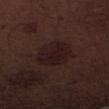Part of a total-body skin-imaging series; this lesion was reviewed on a skin check and was not flagged for biopsy.
A 15 mm close-up tile from a total-body photography series done for melanoma screening.
The total-body-photography lesion software estimated a lesion area of about 14 mm², an outline eccentricity of about 0.65 (0 = round, 1 = elongated), and two-axis asymmetry of about 0.15. It also reported a classifier nevus-likeness of about 25/100 and a detector confidence of about 100 out of 100 that the crop contains a lesion.
The patient is a male aged around 70.
Located on the abdomen.
The tile uses white-light illumination.